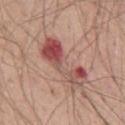Q: Was this lesion biopsied?
A: imaged on a skin check; not biopsied
Q: Lesion size?
A: ≈7.5 mm
Q: How was this image acquired?
A: ~15 mm crop, total-body skin-cancer survey
Q: Lesion location?
A: the front of the torso
Q: What are the patient's age and sex?
A: male, approximately 65 years of age